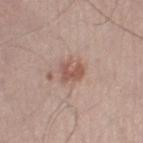TBP lesion metrics = a shape eccentricity near 0.65 and a symmetry-axis asymmetry near 0.3; a nevus-likeness score of about 60/100 and lesion-presence confidence of about 100/100 | lighting = white-light illumination | location = the leg | image source = ~15 mm crop, total-body skin-cancer survey | subject = male, about 55 years old.